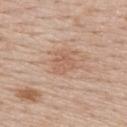Impression:
The lesion was tiled from a total-body skin photograph and was not biopsied.
Context:
From the upper back. This image is a 15 mm lesion crop taken from a total-body photograph. A female subject, roughly 50 years of age. Automated image analysis of the tile measured a footprint of about 5.5 mm² and a symmetry-axis asymmetry near 0.35. The software also gave an average lesion color of about L≈59 a*≈20 b*≈30 (CIELAB). The analysis additionally found border irregularity of about 3 on a 0–10 scale and internal color variation of about 1.5 on a 0–10 scale. The tile uses white-light illumination. Measured at roughly 2.5 mm in maximum diameter.